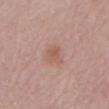This lesion was catalogued during total-body skin photography and was not selected for biopsy. The subject is a female aged around 65. Automated tile analysis of the lesion measured an outline eccentricity of about 0.55 (0 = round, 1 = elongated). It also reported a border-irregularity index near 3/10. The software also gave a lesion-detection confidence of about 100/100. The lesion is on the front of the torso. A close-up tile cropped from a whole-body skin photograph, about 15 mm across.